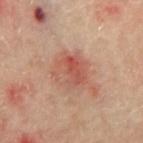Findings:
– workup — no biopsy performed (imaged during a skin exam)
– site — the back
– lesion diameter — ~4 mm (longest diameter)
– image-analysis metrics — a lesion color around L≈53 a*≈25 b*≈29 in CIELAB, roughly 8 lightness units darker than nearby skin, and a lesion-to-skin contrast of about 6 (normalized; higher = more distinct); a nevus-likeness score of about 0/100 and a detector confidence of about 100 out of 100 that the crop contains a lesion
– imaging modality — ~15 mm crop, total-body skin-cancer survey
– patient — male, aged 63–67
– tile lighting — cross-polarized illumination